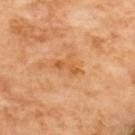Impression:
The lesion was photographed on a routine skin check and not biopsied; there is no pathology result.
Context:
A 15 mm crop from a total-body photograph taken for skin-cancer surveillance. Approximately 3.5 mm at its widest. Imaged with cross-polarized lighting. Automated tile analysis of the lesion measured a lesion area of about 4 mm², an outline eccentricity of about 0.9 (0 = round, 1 = elongated), and a shape-asymmetry score of about 0.35 (0 = symmetric). It also reported internal color variation of about 0 on a 0–10 scale and radial color variation of about 0. And it measured a detector confidence of about 100 out of 100 that the crop contains a lesion. The lesion is located on the upper back.